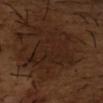Q: Is there a histopathology result?
A: imaged on a skin check; not biopsied
Q: Who is the patient?
A: male, aged approximately 65
Q: What is the lesion's diameter?
A: about 7 mm
Q: Where on the body is the lesion?
A: the right forearm
Q: How was this image acquired?
A: ~15 mm crop, total-body skin-cancer survey